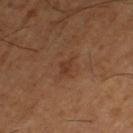Acquisition and patient details:
A male subject, in their mid- to late 60s. Captured under cross-polarized illumination. Cropped from a total-body skin-imaging series; the visible field is about 15 mm. Located on the left thigh. Automated image analysis of the tile measured an area of roughly 3 mm², an eccentricity of roughly 0.85, and a symmetry-axis asymmetry near 0.35. And it measured an average lesion color of about L≈36 a*≈20 b*≈30 (CIELAB), roughly 6 lightness units darker than nearby skin, and a normalized lesion–skin contrast near 5.5. The software also gave internal color variation of about 1 on a 0–10 scale and radial color variation of about 0.5.